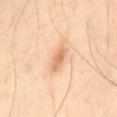{"biopsy_status": "not biopsied; imaged during a skin examination", "site": "front of the torso", "image": {"source": "total-body photography crop", "field_of_view_mm": 15}, "automated_metrics": {"vs_skin_darker_L": 10.0, "vs_skin_contrast_norm": 6.5, "border_irregularity_0_10": 2.5, "color_variation_0_10": 2.0, "peripheral_color_asymmetry": 0.5, "nevus_likeness_0_100": 60, "lesion_detection_confidence_0_100": 100}, "lighting": "cross-polarized", "patient": {"sex": "male", "age_approx": 65}}